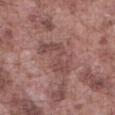| key | value |
|---|---|
| notes | imaged on a skin check; not biopsied |
| lesion diameter | ~5.5 mm (longest diameter) |
| patient | male, aged 73–77 |
| image | ~15 mm tile from a whole-body skin photo |
| lighting | white-light illumination |
| location | the abdomen |
| automated lesion analysis | an automated nevus-likeness rating near 0 out of 100 and a lesion-detection confidence of about 95/100 |